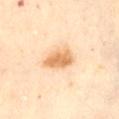Clinical impression: The lesion was photographed on a routine skin check and not biopsied; there is no pathology result. Background: Automated tile analysis of the lesion measured a footprint of about 8.5 mm², an outline eccentricity of about 0.75 (0 = round, 1 = elongated), and a symmetry-axis asymmetry near 0.2. And it measured border irregularity of about 2 on a 0–10 scale, a within-lesion color-variation index near 3.5/10, and a peripheral color-asymmetry measure near 1. From the abdomen. The tile uses cross-polarized illumination. Longest diameter approximately 4 mm. This image is a 15 mm lesion crop taken from a total-body photograph. The patient is a female aged 58 to 62.